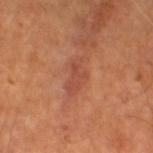- biopsy status: total-body-photography surveillance lesion; no biopsy